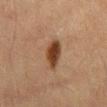Q: Was a biopsy performed?
A: catalogued during a skin exam; not biopsied
Q: Where on the body is the lesion?
A: the abdomen
Q: What is the imaging modality?
A: total-body-photography crop, ~15 mm field of view
Q: What are the patient's age and sex?
A: male, aged 63 to 67